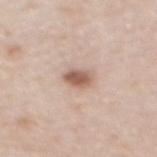This lesion was catalogued during total-body skin photography and was not selected for biopsy.
A lesion tile, about 15 mm wide, cut from a 3D total-body photograph.
The lesion is on the upper back.
The patient is a female aged 48–52.